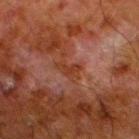Imaged during a routine full-body skin examination; the lesion was not biopsied and no histopathology is available. A 15 mm close-up tile from a total-body photography series done for melanoma screening. The lesion's longest dimension is about 3 mm. From the left lower leg. The lesion-visualizer software estimated an area of roughly 4.5 mm² and a shape-asymmetry score of about 0.4 (0 = symmetric). A male patient, aged 78 to 82. This is a cross-polarized tile.